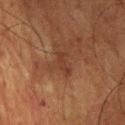Q: Was this lesion biopsied?
A: no biopsy performed (imaged during a skin exam)
Q: How was the tile lit?
A: cross-polarized illumination
Q: Automated lesion metrics?
A: a border-irregularity index near 5/10 and peripheral color asymmetry of about 0; a nevus-likeness score of about 0/100
Q: Lesion location?
A: the chest
Q: Patient demographics?
A: male, aged 58–62
Q: What is the imaging modality?
A: total-body-photography crop, ~15 mm field of view